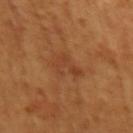Impression: No biopsy was performed on this lesion — it was imaged during a full skin examination and was not determined to be concerning. Image and clinical context: The lesion is on the arm. The subject is a female aged 53 to 57. Approximately 3 mm at its widest. A lesion tile, about 15 mm wide, cut from a 3D total-body photograph.